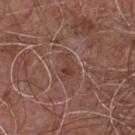automated metrics — an outline eccentricity of about 0.85 (0 = round, 1 = elongated) and two-axis asymmetry of about 0.35; a lesion color around L≈40 a*≈22 b*≈25 in CIELAB and a lesion–skin lightness drop of about 6; a lesion-detection confidence of about 75/100 | anatomic site — the chest | patient — male, aged 53 to 57 | image — 15 mm crop, total-body photography.